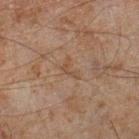biopsy status=imaged on a skin check; not biopsied
size=≈3 mm
lighting=cross-polarized
anatomic site=the right lower leg
image=15 mm crop, total-body photography
patient=male, aged 43–47